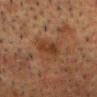Clinical impression: The lesion was photographed on a routine skin check and not biopsied; there is no pathology result. Acquisition and patient details: About 3 mm across. Cropped from a whole-body photographic skin survey; the tile spans about 15 mm. The lesion-visualizer software estimated a footprint of about 5 mm² and a shape eccentricity near 0.8. And it measured an automated nevus-likeness rating near 10 out of 100. A male subject, roughly 60 years of age. Located on the head or neck. Captured under cross-polarized illumination.